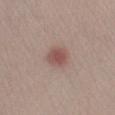site = the left lower leg
subject = female, about 25 years old
image = ~15 mm crop, total-body skin-cancer survey